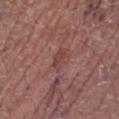No biopsy was performed on this lesion — it was imaged during a full skin examination and was not determined to be concerning.
The lesion-visualizer software estimated a mean CIELAB color near L≈43 a*≈23 b*≈22, a lesion–skin lightness drop of about 7, and a lesion-to-skin contrast of about 5.5 (normalized; higher = more distinct). The software also gave radial color variation of about 0.5. And it measured an automated nevus-likeness rating near 0 out of 100 and a lesion-detection confidence of about 70/100.
A close-up tile cropped from a whole-body skin photograph, about 15 mm across.
A male subject aged approximately 65.
Located on the leg.
The recorded lesion diameter is about 2.5 mm.
Captured under white-light illumination.